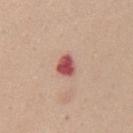{"biopsy_status": "not biopsied; imaged during a skin examination", "image": {"source": "total-body photography crop", "field_of_view_mm": 15}, "site": "mid back", "automated_metrics": {"color_variation_0_10": 3.5, "lesion_detection_confidence_0_100": 100}, "lighting": "white-light", "lesion_size": {"long_diameter_mm_approx": 2.5}, "patient": {"sex": "female", "age_approx": 40}}